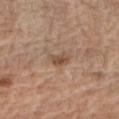Assessment: Imaged during a routine full-body skin examination; the lesion was not biopsied and no histopathology is available. Background: This image is a 15 mm lesion crop taken from a total-body photograph. The patient is a male aged approximately 70. On the right forearm.